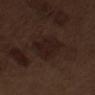Q: Was a biopsy performed?
A: catalogued during a skin exam; not biopsied
Q: How large is the lesion?
A: about 5.5 mm
Q: What is the anatomic site?
A: the arm
Q: What is the imaging modality?
A: ~15 mm crop, total-body skin-cancer survey
Q: What are the patient's age and sex?
A: male, aged around 70
Q: What did automated image analysis measure?
A: a footprint of about 12 mm², an eccentricity of roughly 0.9, and a shape-asymmetry score of about 0.3 (0 = symmetric); a border-irregularity rating of about 3.5/10, internal color variation of about 3 on a 0–10 scale, and a peripheral color-asymmetry measure near 1